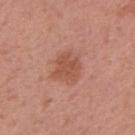Q: Was a biopsy performed?
A: imaged on a skin check; not biopsied
Q: What kind of image is this?
A: 15 mm crop, total-body photography
Q: Where on the body is the lesion?
A: the arm
Q: How large is the lesion?
A: ~3.5 mm (longest diameter)
Q: Illumination type?
A: white-light
Q: What did automated image analysis measure?
A: a footprint of about 7.5 mm², an outline eccentricity of about 0.35 (0 = round, 1 = elongated), and a symmetry-axis asymmetry near 0.25
Q: Who is the patient?
A: female, aged around 50